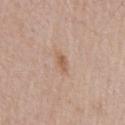Captured during whole-body skin photography for melanoma surveillance; the lesion was not biopsied.
This image is a 15 mm lesion crop taken from a total-body photograph.
An algorithmic analysis of the crop reported a footprint of about 3 mm², a shape eccentricity near 0.85, and a shape-asymmetry score of about 0.25 (0 = symmetric). The analysis additionally found a lesion color around L≈59 a*≈19 b*≈30 in CIELAB, a lesion–skin lightness drop of about 8, and a lesion-to-skin contrast of about 6.5 (normalized; higher = more distinct). It also reported a classifier nevus-likeness of about 5/100 and a detector confidence of about 100 out of 100 that the crop contains a lesion.
The patient is a male approximately 75 years of age.
From the chest.